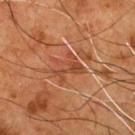Captured during whole-body skin photography for melanoma surveillance; the lesion was not biopsied. The tile uses cross-polarized illumination. Located on the chest. The total-body-photography lesion software estimated an eccentricity of roughly 0.65 and a symmetry-axis asymmetry near 0.35. The analysis additionally found a border-irregularity rating of about 6.5/10, a color-variation rating of about 3.5/10, and a peripheral color-asymmetry measure near 1. A lesion tile, about 15 mm wide, cut from a 3D total-body photograph. The subject is a male in their mid- to late 50s.